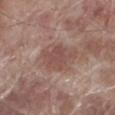follow-up: imaged on a skin check; not biopsied | imaging modality: total-body-photography crop, ~15 mm field of view | lesion size: ≈5 mm | tile lighting: white-light | image-analysis metrics: a lesion area of about 13 mm², an eccentricity of roughly 0.65, and two-axis asymmetry of about 0.25; a lesion color around L≈49 a*≈19 b*≈23 in CIELAB and roughly 8 lightness units darker than nearby skin; a within-lesion color-variation index near 2.5/10 and peripheral color asymmetry of about 1 | anatomic site: the leg | patient: male, roughly 70 years of age.